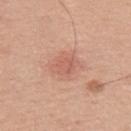The lesion was photographed on a routine skin check and not biopsied; there is no pathology result. The subject is a male aged 43 to 47. Located on the upper back. A 15 mm close-up tile from a total-body photography series done for melanoma screening. Imaged with white-light lighting. About 3.5 mm across.